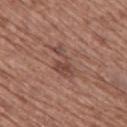Clinical impression:
The lesion was photographed on a routine skin check and not biopsied; there is no pathology result.
Context:
A 15 mm close-up tile from a total-body photography series done for melanoma screening. Longest diameter approximately 4.5 mm. Captured under white-light illumination. The lesion is located on the back. The patient is a female in their mid-70s.